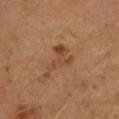Assessment: Captured during whole-body skin photography for melanoma surveillance; the lesion was not biopsied. Acquisition and patient details: The lesion is located on the left upper arm. A 15 mm close-up extracted from a 3D total-body photography capture. The tile uses cross-polarized illumination. A male subject, aged 43–47. Longest diameter approximately 4.5 mm.